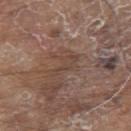Imaged during a routine full-body skin examination; the lesion was not biopsied and no histopathology is available. Longest diameter approximately 4 mm. On the upper back. A male patient, aged around 80. This image is a 15 mm lesion crop taken from a total-body photograph. Captured under white-light illumination.